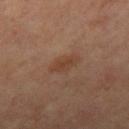The lesion was tiled from a total-body skin photograph and was not biopsied. On the right thigh. The tile uses cross-polarized illumination. Longest diameter approximately 4 mm. A region of skin cropped from a whole-body photographic capture, roughly 15 mm wide. An algorithmic analysis of the crop reported an average lesion color of about L≈40 a*≈19 b*≈28 (CIELAB), about 6 CIELAB-L* units darker than the surrounding skin, and a normalized border contrast of about 6. It also reported a border-irregularity index near 3.5/10 and peripheral color asymmetry of about 0.5. It also reported a classifier nevus-likeness of about 45/100 and a lesion-detection confidence of about 100/100. The subject is a female in their mid-60s.